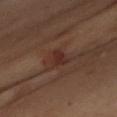Q: Was a biopsy performed?
A: catalogued during a skin exam; not biopsied
Q: What kind of image is this?
A: 15 mm crop, total-body photography
Q: Where on the body is the lesion?
A: the left arm
Q: Automated lesion metrics?
A: a lesion area of about 4 mm², a shape eccentricity near 0.45, and two-axis asymmetry of about 0.35; a lesion color around L≈30 a*≈21 b*≈23 in CIELAB and a normalized border contrast of about 6.5; a border-irregularity index near 3/10, internal color variation of about 2.5 on a 0–10 scale, and radial color variation of about 1; a classifier nevus-likeness of about 15/100 and a detector confidence of about 100 out of 100 that the crop contains a lesion
Q: How large is the lesion?
A: about 2.5 mm
Q: Illumination type?
A: cross-polarized
Q: Who is the patient?
A: female, aged 38–42